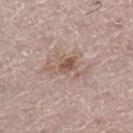Q: Is there a histopathology result?
A: catalogued during a skin exam; not biopsied
Q: What is the imaging modality?
A: ~15 mm tile from a whole-body skin photo
Q: Lesion size?
A: ~5.5 mm (longest diameter)
Q: Lesion location?
A: the left leg
Q: Patient demographics?
A: male, about 70 years old
Q: How was the tile lit?
A: white-light